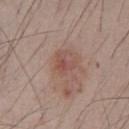The lesion was tiled from a total-body skin photograph and was not biopsied.
A lesion tile, about 15 mm wide, cut from a 3D total-body photograph.
A male subject, approximately 40 years of age.
Captured under white-light illumination.
The lesion is located on the chest.
Measured at roughly 3 mm in maximum diameter.